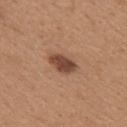Imaged during a routine full-body skin examination; the lesion was not biopsied and no histopathology is available. A roughly 15 mm field-of-view crop from a total-body skin photograph. The lesion is on the upper back. The subject is a female aged 28–32.